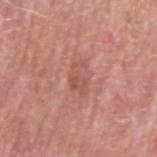Clinical impression:
The lesion was photographed on a routine skin check and not biopsied; there is no pathology result.
Background:
The subject is a male in their mid- to late 60s. About 3.5 mm across. Automated tile analysis of the lesion measured a mean CIELAB color near L≈54 a*≈26 b*≈28 and a normalized lesion–skin contrast near 5.5. It also reported a border-irregularity rating of about 6.5/10 and peripheral color asymmetry of about 0.5. The software also gave a nevus-likeness score of about 0/100 and lesion-presence confidence of about 100/100. This is a white-light tile. From the arm. A region of skin cropped from a whole-body photographic capture, roughly 15 mm wide.